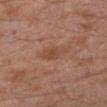Captured during whole-body skin photography for melanoma surveillance; the lesion was not biopsied. Measured at roughly 2.5 mm in maximum diameter. This is a cross-polarized tile. A male subject aged around 30. Automated image analysis of the tile measured an area of roughly 5 mm², a shape eccentricity near 0.55, and two-axis asymmetry of about 0.25. The software also gave a lesion-detection confidence of about 100/100. A close-up tile cropped from a whole-body skin photograph, about 15 mm across. The lesion is located on the right forearm.